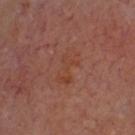Q: Was a biopsy performed?
A: imaged on a skin check; not biopsied
Q: What lighting was used for the tile?
A: cross-polarized
Q: What is the anatomic site?
A: the head or neck
Q: What kind of image is this?
A: ~15 mm crop, total-body skin-cancer survey
Q: Patient demographics?
A: in their mid- to late 60s